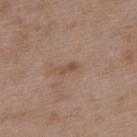Assessment:
The lesion was tiled from a total-body skin photograph and was not biopsied.
Acquisition and patient details:
Approximately 2.5 mm at its widest. The lesion is on the upper back. A region of skin cropped from a whole-body photographic capture, roughly 15 mm wide. A male subject approximately 50 years of age. Imaged with white-light lighting.